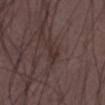workup: no biopsy performed (imaged during a skin exam).